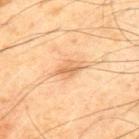Notes:
– workup · imaged on a skin check; not biopsied
– patient · male, roughly 70 years of age
– lighting · cross-polarized illumination
– image · total-body-photography crop, ~15 mm field of view
– diameter · about 3.5 mm
– site · the chest